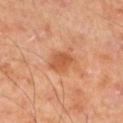follow-up=total-body-photography surveillance lesion; no biopsy
patient=male, roughly 70 years of age
tile lighting=cross-polarized illumination
image source=15 mm crop, total-body photography
site=the leg
size=about 3 mm